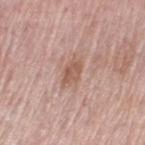| key | value |
|---|---|
| image source | 15 mm crop, total-body photography |
| TBP lesion metrics | radial color variation of about 1 |
| illumination | white-light illumination |
| site | the left upper arm |
| subject | female, about 60 years old |